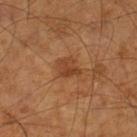Impression:
The lesion was tiled from a total-body skin photograph and was not biopsied.
Background:
From the left lower leg. Captured under cross-polarized illumination. A male subject aged around 65. A region of skin cropped from a whole-body photographic capture, roughly 15 mm wide.